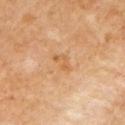Findings:
* biopsy status — no biopsy performed (imaged during a skin exam)
* subject — female, in their 50s
* location — the left upper arm
* acquisition — ~15 mm tile from a whole-body skin photo
* lesion size — about 3 mm
* tile lighting — cross-polarized illumination